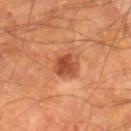Impression:
The lesion was photographed on a routine skin check and not biopsied; there is no pathology result.
Context:
A male patient, approximately 65 years of age. From the right lower leg. A close-up tile cropped from a whole-body skin photograph, about 15 mm across. Automated tile analysis of the lesion measured border irregularity of about 2 on a 0–10 scale and radial color variation of about 1. The analysis additionally found an automated nevus-likeness rating near 80 out of 100 and a detector confidence of about 100 out of 100 that the crop contains a lesion.